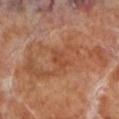| feature | finding |
|---|---|
| workup | total-body-photography surveillance lesion; no biopsy |
| imaging modality | ~15 mm crop, total-body skin-cancer survey |
| site | the left lower leg |
| lighting | cross-polarized illumination |
| subject | male, aged 68 to 72 |
| size | ≈3 mm |